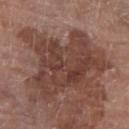Q: Is there a histopathology result?
A: total-body-photography surveillance lesion; no biopsy
Q: Lesion size?
A: ~9 mm (longest diameter)
Q: Automated lesion metrics?
A: a footprint of about 36 mm², a shape eccentricity near 0.75, and a symmetry-axis asymmetry near 0.4; a mean CIELAB color near L≈40 a*≈20 b*≈23, roughly 9 lightness units darker than nearby skin, and a normalized lesion–skin contrast near 7.5; a nevus-likeness score of about 0/100 and a detector confidence of about 85 out of 100 that the crop contains a lesion
Q: What is the anatomic site?
A: the right lower leg
Q: What are the patient's age and sex?
A: female, about 80 years old
Q: What is the imaging modality?
A: total-body-photography crop, ~15 mm field of view
Q: What lighting was used for the tile?
A: white-light illumination